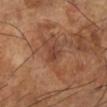No biopsy was performed on this lesion — it was imaged during a full skin examination and was not determined to be concerning. Located on the left lower leg. The tile uses cross-polarized illumination. The lesion-visualizer software estimated an outline eccentricity of about 0.75 (0 = round, 1 = elongated) and a shape-asymmetry score of about 0.35 (0 = symmetric). The analysis additionally found a mean CIELAB color near L≈41 a*≈23 b*≈29 and a lesion-to-skin contrast of about 6 (normalized; higher = more distinct). And it measured a border-irregularity rating of about 3/10, a within-lesion color-variation index near 2/10, and peripheral color asymmetry of about 0.5. A close-up tile cropped from a whole-body skin photograph, about 15 mm across. Measured at roughly 2.5 mm in maximum diameter. A male subject, aged approximately 65.